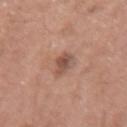biopsy status: catalogued during a skin exam; not biopsied | illumination: white-light | site: the right forearm | acquisition: 15 mm crop, total-body photography | patient: female, aged around 40 | diameter: about 3 mm.